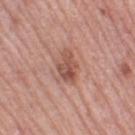Notes:
* follow-up — no biopsy performed (imaged during a skin exam)
* patient — female, aged approximately 65
* acquisition — total-body-photography crop, ~15 mm field of view
* tile lighting — white-light
* automated lesion analysis — an area of roughly 6 mm², an outline eccentricity of about 0.8 (0 = round, 1 = elongated), and two-axis asymmetry of about 0.35; a border-irregularity rating of about 3.5/10, a color-variation rating of about 5/10, and radial color variation of about 1.5; a nevus-likeness score of about 30/100 and a detector confidence of about 100 out of 100 that the crop contains a lesion
* anatomic site — the left thigh